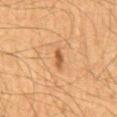notes — no biopsy performed (imaged during a skin exam)
image source — ~15 mm crop, total-body skin-cancer survey
illumination — cross-polarized illumination
TBP lesion metrics — about 10 CIELAB-L* units darker than the surrounding skin and a normalized border contrast of about 8; a peripheral color-asymmetry measure near 0; a nevus-likeness score of about 80/100
diameter — about 2.5 mm
subject — male, approximately 65 years of age
anatomic site — the back